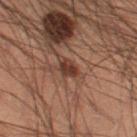biopsy_status: not biopsied; imaged during a skin examination
site: left thigh
lighting: cross-polarized
patient:
  sex: male
  age_approx: 55
image:
  source: total-body photography crop
  field_of_view_mm: 15
automated_metrics:
  area_mm2_approx: 3.0
  eccentricity: 0.85
  shape_asymmetry: 0.25
  cielab_L: 28
  cielab_a: 17
  cielab_b: 22
  vs_skin_darker_L: 10.0
  vs_skin_contrast_norm: 10.5
  border_irregularity_0_10: 2.5
  color_variation_0_10: 1.0
  nevus_likeness_0_100: 100
  lesion_detection_confidence_0_100: 100
lesion_size:
  long_diameter_mm_approx: 2.5